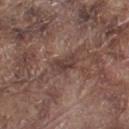{"biopsy_status": "not biopsied; imaged during a skin examination", "site": "left thigh", "image": {"source": "total-body photography crop", "field_of_view_mm": 15}, "patient": {"sex": "male", "age_approx": 75}, "automated_metrics": {"cielab_L": 39, "cielab_a": 17, "cielab_b": 21, "vs_skin_darker_L": 8.0, "border_irregularity_0_10": 3.5, "color_variation_0_10": 2.0, "peripheral_color_asymmetry": 1.0}, "lighting": "white-light"}